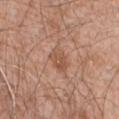{"biopsy_status": "not biopsied; imaged during a skin examination", "lesion_size": {"long_diameter_mm_approx": 3.0}, "lighting": "white-light", "patient": {"sex": "male", "age_approx": 60}, "image": {"source": "total-body photography crop", "field_of_view_mm": 15}, "site": "lower back"}